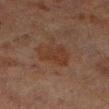The lesion was photographed on a routine skin check and not biopsied; there is no pathology result. This is a cross-polarized tile. Longest diameter approximately 4 mm. Cropped from a total-body skin-imaging series; the visible field is about 15 mm. From the leg. A male patient, roughly 70 years of age.